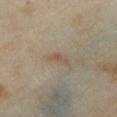The lesion was tiled from a total-body skin photograph and was not biopsied. An algorithmic analysis of the crop reported a lesion area of about 2.5 mm², a shape eccentricity near 0.9, and a symmetry-axis asymmetry near 0.4. And it measured a border-irregularity index near 4/10, a color-variation rating of about 0/10, and a peripheral color-asymmetry measure near 0. Cropped from a total-body skin-imaging series; the visible field is about 15 mm. The lesion is on the chest. Captured under cross-polarized illumination. The patient is a female aged 33–37.